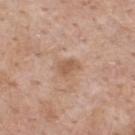No biopsy was performed on this lesion — it was imaged during a full skin examination and was not determined to be concerning.
A male subject aged around 60.
This is a white-light tile.
The recorded lesion diameter is about 2.5 mm.
On the back.
Cropped from a total-body skin-imaging series; the visible field is about 15 mm.